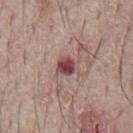workup = imaged on a skin check; not biopsied | diameter = about 2.5 mm | image source = ~15 mm crop, total-body skin-cancer survey | image-analysis metrics = an area of roughly 4.5 mm², an eccentricity of roughly 0.45, and a symmetry-axis asymmetry near 0.2; a normalized border contrast of about 11; internal color variation of about 3.5 on a 0–10 scale and a peripheral color-asymmetry measure near 1.5 | subject = male, aged approximately 65 | tile lighting = white-light | anatomic site = the front of the torso.